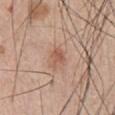Clinical impression:
No biopsy was performed on this lesion — it was imaged during a full skin examination and was not determined to be concerning.
Acquisition and patient details:
A male subject aged approximately 65. This is a white-light tile. The lesion is located on the chest. The lesion-visualizer software estimated a lesion color around L≈56 a*≈22 b*≈29 in CIELAB, a lesion–skin lightness drop of about 9, and a lesion-to-skin contrast of about 6.5 (normalized; higher = more distinct). The software also gave border irregularity of about 2.5 on a 0–10 scale, internal color variation of about 3 on a 0–10 scale, and peripheral color asymmetry of about 1. A 15 mm crop from a total-body photograph taken for skin-cancer surveillance. The recorded lesion diameter is about 2.5 mm.